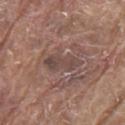Clinical impression: No biopsy was performed on this lesion — it was imaged during a full skin examination and was not determined to be concerning. Context: The lesion is on the chest. A region of skin cropped from a whole-body photographic capture, roughly 15 mm wide. The patient is a male about 80 years old. Automated tile analysis of the lesion measured a lesion area of about 8.5 mm², a shape eccentricity near 0.85, and two-axis asymmetry of about 0.25. And it measured a mean CIELAB color near L≈45 a*≈16 b*≈21, a lesion–skin lightness drop of about 7, and a normalized lesion–skin contrast near 6. And it measured a within-lesion color-variation index near 3.5/10 and radial color variation of about 1. The analysis additionally found a detector confidence of about 85 out of 100 that the crop contains a lesion. About 5 mm across. This is a white-light tile.